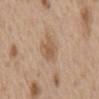notes: imaged on a skin check; not biopsied
size: ≈3 mm
location: the back
imaging modality: ~15 mm crop, total-body skin-cancer survey
lighting: white-light illumination
patient: male, approximately 70 years of age